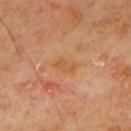Assessment: This lesion was catalogued during total-body skin photography and was not selected for biopsy. Context: On the back. Imaged with cross-polarized lighting. A male patient approximately 70 years of age. Cropped from a whole-body photographic skin survey; the tile spans about 15 mm. The lesion's longest dimension is about 3.5 mm.